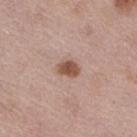A female subject, aged around 40. Imaged with white-light lighting. A 15 mm close-up tile from a total-body photography series done for melanoma screening. The lesion is located on the right thigh. About 2.5 mm across.